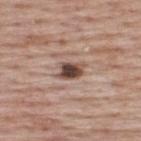Q: Is there a histopathology result?
A: no biopsy performed (imaged during a skin exam)
Q: Lesion size?
A: about 3.5 mm
Q: Lesion location?
A: the upper back
Q: What kind of image is this?
A: total-body-photography crop, ~15 mm field of view
Q: Who is the patient?
A: male, in their mid-60s
Q: Automated lesion metrics?
A: a footprint of about 5.5 mm², an outline eccentricity of about 0.8 (0 = round, 1 = elongated), and a symmetry-axis asymmetry near 0.2; a mean CIELAB color near L≈47 a*≈18 b*≈24 and a lesion–skin lightness drop of about 17; a border-irregularity rating of about 2/10 and a peripheral color-asymmetry measure near 1.5; an automated nevus-likeness rating near 100 out of 100 and a lesion-detection confidence of about 100/100
Q: How was the tile lit?
A: white-light illumination